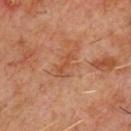Notes:
• workup — no biopsy performed (imaged during a skin exam)
• lesion size — about 3 mm
• image-analysis metrics — an area of roughly 2.5 mm² and an outline eccentricity of about 0.95 (0 = round, 1 = elongated); a mean CIELAB color near L≈46 a*≈25 b*≈33, roughly 7 lightness units darker than nearby skin, and a lesion-to-skin contrast of about 5.5 (normalized; higher = more distinct); radial color variation of about 0; a classifier nevus-likeness of about 0/100 and a detector confidence of about 100 out of 100 that the crop contains a lesion
• body site — the chest
• image — ~15 mm crop, total-body skin-cancer survey
• subject — male, aged around 60